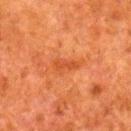illumination: cross-polarized | image-analysis metrics: a lesion area of about 4 mm², a shape eccentricity near 0.95, and a shape-asymmetry score of about 0.35 (0 = symmetric); a mean CIELAB color near L≈42 a*≈30 b*≈38, about 7 CIELAB-L* units darker than the surrounding skin, and a lesion-to-skin contrast of about 5.5 (normalized; higher = more distinct); border irregularity of about 4 on a 0–10 scale, a color-variation rating of about 1.5/10, and radial color variation of about 0.5 | site: the right lower leg | acquisition: ~15 mm tile from a whole-body skin photo | lesion diameter: about 3.5 mm | patient: male, aged around 80.